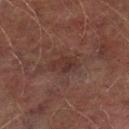Captured during whole-body skin photography for melanoma surveillance; the lesion was not biopsied. On the left lower leg. About 3 mm across. A male patient, aged 73–77. Automated image analysis of the tile measured an area of roughly 5 mm², an eccentricity of roughly 0.8, and a shape-asymmetry score of about 0.25 (0 = symmetric). The software also gave a border-irregularity rating of about 2.5/10, internal color variation of about 2 on a 0–10 scale, and a peripheral color-asymmetry measure near 0.5. A 15 mm close-up tile from a total-body photography series done for melanoma screening. Captured under cross-polarized illumination.